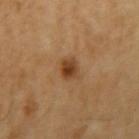Measured at roughly 2.5 mm in maximum diameter. A 15 mm close-up extracted from a 3D total-body photography capture. The subject is a male approximately 65 years of age. This is a cross-polarized tile. From the right upper arm.